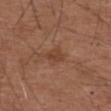{
  "biopsy_status": "not biopsied; imaged during a skin examination",
  "lighting": "white-light",
  "lesion_size": {
    "long_diameter_mm_approx": 2.5
  },
  "image": {
    "source": "total-body photography crop",
    "field_of_view_mm": 15
  },
  "patient": {
    "sex": "male",
    "age_approx": 75
  },
  "site": "upper back"
}